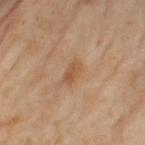biopsy status = catalogued during a skin exam; not biopsied
body site = the left upper arm
imaging modality = ~15 mm tile from a whole-body skin photo
lesion diameter = ~2.5 mm (longest diameter)
subject = female, aged approximately 55